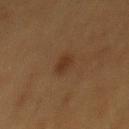{"biopsy_status": "not biopsied; imaged during a skin examination", "lesion_size": {"long_diameter_mm_approx": 3.0}, "patient": {"sex": "male", "age_approx": 55}, "automated_metrics": {"area_mm2_approx": 4.0, "cielab_L": 29, "cielab_a": 16, "cielab_b": 28, "vs_skin_darker_L": 6.0, "border_irregularity_0_10": 2.5, "color_variation_0_10": 1.5, "peripheral_color_asymmetry": 0.5, "nevus_likeness_0_100": 95, "lesion_detection_confidence_0_100": 100}, "image": {"source": "total-body photography crop", "field_of_view_mm": 15}, "lighting": "cross-polarized", "site": "chest"}